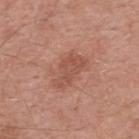Part of a total-body skin-imaging series; this lesion was reviewed on a skin check and was not flagged for biopsy. A 15 mm crop from a total-body photograph taken for skin-cancer surveillance. The patient is a male roughly 55 years of age. The lesion's longest dimension is about 4.5 mm. Automated image analysis of the tile measured a lesion area of about 8.5 mm² and an eccentricity of roughly 0.85. And it measured a mean CIELAB color near L≈52 a*≈25 b*≈29 and about 8 CIELAB-L* units darker than the surrounding skin. And it measured a border-irregularity index near 4/10, internal color variation of about 2.5 on a 0–10 scale, and radial color variation of about 0.5. And it measured an automated nevus-likeness rating near 5 out of 100 and a detector confidence of about 100 out of 100 that the crop contains a lesion. The lesion is on the upper back.